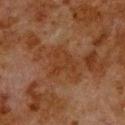Clinical impression:
Part of a total-body skin-imaging series; this lesion was reviewed on a skin check and was not flagged for biopsy.
Background:
Cropped from a whole-body photographic skin survey; the tile spans about 15 mm. Captured under cross-polarized illumination. The lesion is on the upper back. The lesion's longest dimension is about 3.5 mm. A male subject aged 78–82.